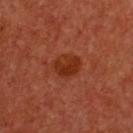The lesion was tiled from a total-body skin photograph and was not biopsied.
Approximately 3.5 mm at its widest.
The patient is a female about 50 years old.
Cropped from a whole-body photographic skin survey; the tile spans about 15 mm.
The tile uses cross-polarized illumination.